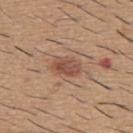follow-up = catalogued during a skin exam; not biopsied | patient = male, aged around 60 | image = ~15 mm crop, total-body skin-cancer survey | lighting = white-light | location = the back.